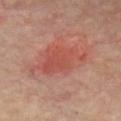• notes · imaged on a skin check; not biopsied
• image-analysis metrics · a border-irregularity index near 5/10, internal color variation of about 3 on a 0–10 scale, and radial color variation of about 1; a classifier nevus-likeness of about 100/100 and lesion-presence confidence of about 100/100
• illumination · cross-polarized
• lesion diameter · ≈6 mm
• subject · female, aged around 50
• acquisition · 15 mm crop, total-body photography
• location · the front of the torso